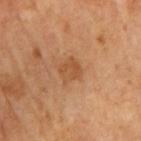Imaged during a routine full-body skin examination; the lesion was not biopsied and no histopathology is available.
The recorded lesion diameter is about 2.5 mm.
This is a cross-polarized tile.
The lesion is located on the right upper arm.
Cropped from a whole-body photographic skin survey; the tile spans about 15 mm.
An algorithmic analysis of the crop reported a footprint of about 5.5 mm², an eccentricity of roughly 0.2, and a symmetry-axis asymmetry near 0.3. And it measured a lesion–skin lightness drop of about 7 and a lesion-to-skin contrast of about 6 (normalized; higher = more distinct).
The patient is a female roughly 70 years of age.